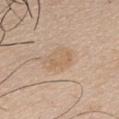Q: Was a biopsy performed?
A: total-body-photography surveillance lesion; no biopsy
Q: Lesion size?
A: about 3.5 mm
Q: What kind of image is this?
A: total-body-photography crop, ~15 mm field of view
Q: Illumination type?
A: white-light illumination
Q: What are the patient's age and sex?
A: male, aged 43 to 47
Q: Lesion location?
A: the chest